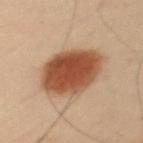size: ~7.5 mm (longest diameter); site: the left upper arm; patient: male, about 55 years old; imaging modality: total-body-photography crop, ~15 mm field of view.